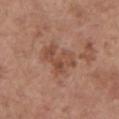| key | value |
|---|---|
| follow-up | imaged on a skin check; not biopsied |
| anatomic site | the left upper arm |
| tile lighting | white-light illumination |
| acquisition | ~15 mm crop, total-body skin-cancer survey |
| subject | female, in their mid-60s |
| diameter | ~4.5 mm (longest diameter) |